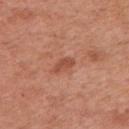Acquisition and patient details: The subject is a female in their 50s. A 15 mm close-up extracted from a 3D total-body photography capture. Located on the left upper arm. The tile uses white-light illumination. The recorded lesion diameter is about 2.5 mm. The total-body-photography lesion software estimated an average lesion color of about L≈50 a*≈28 b*≈32 (CIELAB) and about 9 CIELAB-L* units darker than the surrounding skin. The software also gave a lesion-detection confidence of about 100/100.